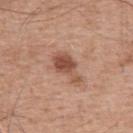Clinical impression: Imaged during a routine full-body skin examination; the lesion was not biopsied and no histopathology is available. Background: A male subject in their mid-50s. Imaged with white-light lighting. Located on the upper back. This image is a 15 mm lesion crop taken from a total-body photograph. An algorithmic analysis of the crop reported a lesion area of about 7 mm², a shape eccentricity near 0.85, and two-axis asymmetry of about 0.4. The software also gave a mean CIELAB color near L≈51 a*≈23 b*≈29, roughly 12 lightness units darker than nearby skin, and a normalized lesion–skin contrast near 8.5.